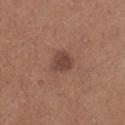Clinical impression: Imaged during a routine full-body skin examination; the lesion was not biopsied and no histopathology is available. Acquisition and patient details: From the left lower leg. A roughly 15 mm field-of-view crop from a total-body skin photograph. A male patient aged 63–67. The tile uses white-light illumination. About 3 mm across.